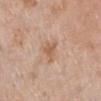Imaged during a routine full-body skin examination; the lesion was not biopsied and no histopathology is available. The lesion is on the right lower leg. Captured under white-light illumination. The total-body-photography lesion software estimated a footprint of about 4.5 mm², a shape eccentricity near 0.65, and two-axis asymmetry of about 0.45. The analysis additionally found roughly 8 lightness units darker than nearby skin and a normalized lesion–skin contrast near 6. And it measured border irregularity of about 4 on a 0–10 scale and internal color variation of about 2 on a 0–10 scale. The software also gave a classifier nevus-likeness of about 0/100 and a detector confidence of about 100 out of 100 that the crop contains a lesion. The patient is a female aged 68 to 72. This image is a 15 mm lesion crop taken from a total-body photograph.